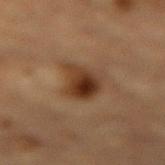No biopsy was performed on this lesion — it was imaged during a full skin examination and was not determined to be concerning. A male patient in their mid-80s or older. Imaged with cross-polarized lighting. The lesion-visualizer software estimated a footprint of about 12 mm², an eccentricity of roughly 0.65, and two-axis asymmetry of about 0.35. It also reported a nevus-likeness score of about 100/100 and a detector confidence of about 100 out of 100 that the crop contains a lesion. On the lower back. Longest diameter approximately 4.5 mm. Cropped from a total-body skin-imaging series; the visible field is about 15 mm.